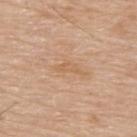No biopsy was performed on this lesion — it was imaged during a full skin examination and was not determined to be concerning.
A male subject aged approximately 80.
Automated image analysis of the tile measured an area of roughly 3 mm² and an outline eccentricity of about 0.9 (0 = round, 1 = elongated). And it measured an average lesion color of about L≈61 a*≈19 b*≈35 (CIELAB), a lesion–skin lightness drop of about 6, and a normalized lesion–skin contrast near 4.5. And it measured a border-irregularity rating of about 4.5/10 and a within-lesion color-variation index near 0/10.
A region of skin cropped from a whole-body photographic capture, roughly 15 mm wide.
From the upper back.